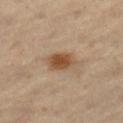Captured during whole-body skin photography for melanoma surveillance; the lesion was not biopsied.
Measured at roughly 3.5 mm in maximum diameter.
The lesion is located on the left thigh.
A male patient roughly 60 years of age.
Captured under cross-polarized illumination.
This image is a 15 mm lesion crop taken from a total-body photograph.